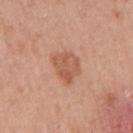No biopsy was performed on this lesion — it was imaged during a full skin examination and was not determined to be concerning. The patient is a female roughly 55 years of age. Approximately 3.5 mm at its widest. On the right upper arm. This image is a 15 mm lesion crop taken from a total-body photograph.